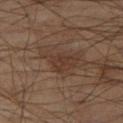{"biopsy_status": "not biopsied; imaged during a skin examination", "patient": {"sex": "male", "age_approx": 55}, "lighting": "cross-polarized", "lesion_size": {"long_diameter_mm_approx": 3.0}, "site": "right thigh", "image": {"source": "total-body photography crop", "field_of_view_mm": 15}, "automated_metrics": {"area_mm2_approx": 4.0, "eccentricity": 0.75, "shape_asymmetry": 0.5, "color_variation_0_10": 1.5}}